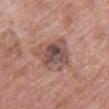Case summary:
* biopsy status — no biopsy performed (imaged during a skin exam)
* image source — ~15 mm tile from a whole-body skin photo
* automated metrics — a lesion area of about 14 mm², an eccentricity of roughly 0.5, and a shape-asymmetry score of about 0.25 (0 = symmetric); border irregularity of about 3.5 on a 0–10 scale, a within-lesion color-variation index near 8/10, and peripheral color asymmetry of about 2.5; an automated nevus-likeness rating near 10 out of 100 and a detector confidence of about 100 out of 100 that the crop contains a lesion
* lighting — white-light
* site — the chest
* subject — male, aged approximately 85 or older
* size — about 5 mm